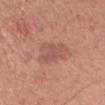No biopsy was performed on this lesion — it was imaged during a full skin examination and was not determined to be concerning.
Cropped from a total-body skin-imaging series; the visible field is about 15 mm.
The subject is a female roughly 55 years of age.
The lesion is on the head or neck.
The lesion-visualizer software estimated a border-irregularity index near 3/10. And it measured an automated nevus-likeness rating near 0 out of 100 and lesion-presence confidence of about 100/100.